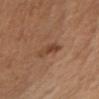Q: Was a biopsy performed?
A: no biopsy performed (imaged during a skin exam)
Q: What is the anatomic site?
A: the right upper arm
Q: Who is the patient?
A: female, in their 40s
Q: What did automated image analysis measure?
A: border irregularity of about 3.5 on a 0–10 scale and internal color variation of about 4 on a 0–10 scale
Q: How large is the lesion?
A: ~2.5 mm (longest diameter)
Q: What is the imaging modality?
A: total-body-photography crop, ~15 mm field of view
Q: What lighting was used for the tile?
A: cross-polarized illumination